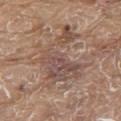{
  "biopsy_status": "not biopsied; imaged during a skin examination",
  "patient": {
    "sex": "male",
    "age_approx": 80
  },
  "site": "back",
  "image": {
    "source": "total-body photography crop",
    "field_of_view_mm": 15
  }
}